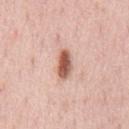Background:
Captured under white-light illumination. The patient is a male aged 38–42. This image is a 15 mm lesion crop taken from a total-body photograph. The lesion's longest dimension is about 3.5 mm. An algorithmic analysis of the crop reported a lesion color around L≈59 a*≈23 b*≈29 in CIELAB, a lesion–skin lightness drop of about 17, and a normalized border contrast of about 10.5. It also reported a border-irregularity rating of about 2.5/10, a color-variation rating of about 5.5/10, and peripheral color asymmetry of about 1.5. It also reported a classifier nevus-likeness of about 100/100 and a detector confidence of about 100 out of 100 that the crop contains a lesion. Located on the chest.